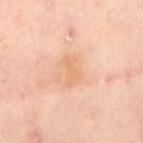biopsy status: imaged on a skin check; not biopsied | anatomic site: the right thigh | subject: female, aged around 55 | diameter: about 3.5 mm | image: total-body-photography crop, ~15 mm field of view.